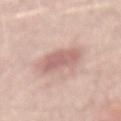notes: total-body-photography surveillance lesion; no biopsy | automated metrics: an area of roughly 12 mm² and a shape-asymmetry score of about 0.2 (0 = symmetric); an average lesion color of about L≈63 a*≈21 b*≈23 (CIELAB) and a normalized lesion–skin contrast near 6.5; a border-irregularity index near 2.5/10, a within-lesion color-variation index near 2.5/10, and radial color variation of about 1 | subject: male, aged around 70 | image source: total-body-photography crop, ~15 mm field of view | lesion size: ~5.5 mm (longest diameter) | body site: the back | tile lighting: white-light illumination.